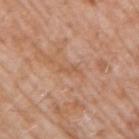No biopsy was performed on this lesion — it was imaged during a full skin examination and was not determined to be concerning.
The lesion is on the arm.
The patient is a male aged 58 to 62.
About 2.5 mm across.
This image is a 15 mm lesion crop taken from a total-body photograph.